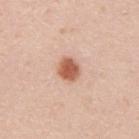{"biopsy_status": "not biopsied; imaged during a skin examination", "patient": {"sex": "male", "age_approx": 35}, "image": {"source": "total-body photography crop", "field_of_view_mm": 15}, "lesion_size": {"long_diameter_mm_approx": 3.0}, "lighting": "white-light", "site": "upper back", "automated_metrics": {"area_mm2_approx": 6.0, "eccentricity": 0.55, "cielab_L": 60, "cielab_a": 25, "cielab_b": 32, "vs_skin_darker_L": 15.0, "vs_skin_contrast_norm": 9.5, "border_irregularity_0_10": 1.0, "color_variation_0_10": 3.5, "peripheral_color_asymmetry": 1.0}}